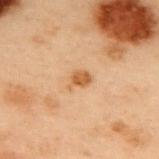No biopsy was performed on this lesion — it was imaged during a full skin examination and was not determined to be concerning. Imaged with cross-polarized lighting. The patient is a male aged approximately 55. On the upper back. The recorded lesion diameter is about 2.5 mm. A roughly 15 mm field-of-view crop from a total-body skin photograph.